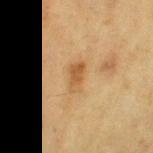follow-up: catalogued during a skin exam; not biopsied
size: ~3.5 mm (longest diameter)
lighting: cross-polarized illumination
subject: female, in their mid- to late 50s
site: the left thigh
image: ~15 mm tile from a whole-body skin photo